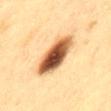Clinical impression: Recorded during total-body skin imaging; not selected for excision or biopsy. Background: Located on the lower back. Imaged with cross-polarized lighting. A 15 mm close-up tile from a total-body photography series done for melanoma screening. About 6 mm across. The patient is a female aged around 40.